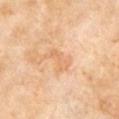subject — male, aged 68–72 | tile lighting — cross-polarized illumination | size — ≈3 mm | image — ~15 mm crop, total-body skin-cancer survey | site — the chest | automated metrics — a lesion area of about 3.5 mm², an eccentricity of roughly 0.95, and two-axis asymmetry of about 0.35.